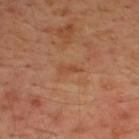biopsy status: no biopsy performed (imaged during a skin exam); image source: total-body-photography crop, ~15 mm field of view; anatomic site: the upper back; subject: male, aged 43 to 47.